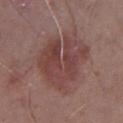Recorded during total-body skin imaging; not selected for excision or biopsy.
On the left lower leg.
A male subject, approximately 65 years of age.
A 15 mm crop from a total-body photograph taken for skin-cancer surveillance.
Longest diameter approximately 6.5 mm.
The tile uses white-light illumination.